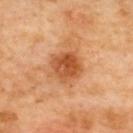Captured during whole-body skin photography for melanoma surveillance; the lesion was not biopsied.